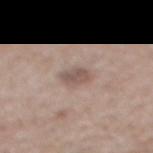* workup — catalogued during a skin exam; not biopsied
* image source — ~15 mm crop, total-body skin-cancer survey
* TBP lesion metrics — a lesion area of about 5 mm², an outline eccentricity of about 0.65 (0 = round, 1 = elongated), and two-axis asymmetry of about 0.2
* patient — male, in their mid-50s
* site — the mid back
* size — ≈2.5 mm
* tile lighting — white-light illumination